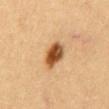Clinical impression:
The lesion was photographed on a routine skin check and not biopsied; there is no pathology result.
Background:
A female patient, roughly 40 years of age. A 15 mm close-up tile from a total-body photography series done for melanoma screening. The lesion-visualizer software estimated a lesion area of about 7.5 mm². The software also gave a lesion color around L≈43 a*≈19 b*≈34 in CIELAB and a lesion–skin lightness drop of about 16. The analysis additionally found a border-irregularity index near 2/10 and a peripheral color-asymmetry measure near 2. The lesion is on the mid back.